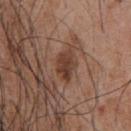Q: Is there a histopathology result?
A: imaged on a skin check; not biopsied
Q: How was the tile lit?
A: white-light
Q: What is the imaging modality?
A: ~15 mm crop, total-body skin-cancer survey
Q: What did automated image analysis measure?
A: a lesion color around L≈39 a*≈20 b*≈26 in CIELAB, roughly 10 lightness units darker than nearby skin, and a normalized border contrast of about 8; a border-irregularity rating of about 3/10 and a within-lesion color-variation index near 3.5/10; a classifier nevus-likeness of about 60/100
Q: What is the lesion's diameter?
A: ≈3.5 mm
Q: What are the patient's age and sex?
A: male, in their mid-50s
Q: Where on the body is the lesion?
A: the chest